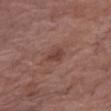workup: no biopsy performed (imaged during a skin exam) | patient: male, in their 80s | automated metrics: a footprint of about 3.5 mm², an outline eccentricity of about 0.8 (0 = round, 1 = elongated), and two-axis asymmetry of about 0.3; a lesion color around L≈41 a*≈22 b*≈23 in CIELAB, about 8 CIELAB-L* units darker than the surrounding skin, and a normalized lesion–skin contrast near 7; border irregularity of about 3 on a 0–10 scale and radial color variation of about 0.5; a classifier nevus-likeness of about 20/100 and a detector confidence of about 100 out of 100 that the crop contains a lesion | image: 15 mm crop, total-body photography | body site: the right thigh | diameter: ~3 mm (longest diameter) | lighting: white-light.